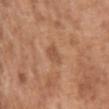Case summary:
– workup · total-body-photography surveillance lesion; no biopsy
– patient · male, aged 63–67
– site · the chest
– illumination · white-light illumination
– diameter · ≈2.5 mm
– imaging modality · total-body-photography crop, ~15 mm field of view